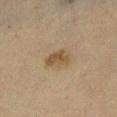The lesion was photographed on a routine skin check and not biopsied; there is no pathology result. The tile uses cross-polarized illumination. Measured at roughly 3.5 mm in maximum diameter. A female subject, approximately 55 years of age. A roughly 15 mm field-of-view crop from a total-body skin photograph. From the left lower leg.